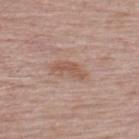No biopsy was performed on this lesion — it was imaged during a full skin examination and was not determined to be concerning.
The lesion is on the upper back.
The patient is a male in their mid-60s.
A lesion tile, about 15 mm wide, cut from a 3D total-body photograph.
Imaged with white-light lighting.
An algorithmic analysis of the crop reported an area of roughly 4.5 mm², an outline eccentricity of about 0.9 (0 = round, 1 = elongated), and a symmetry-axis asymmetry near 0.5. And it measured a border-irregularity index near 5.5/10 and a within-lesion color-variation index near 0.5/10.